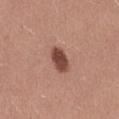  biopsy_status: not biopsied; imaged during a skin examination
  image:
    source: total-body photography crop
    field_of_view_mm: 15
  site: left thigh
  lesion_size:
    long_diameter_mm_approx: 3.5
  patient:
    sex: female
    age_approx: 25
  lighting: white-light